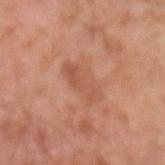Findings:
* biopsy status — catalogued during a skin exam; not biopsied
* TBP lesion metrics — an area of roughly 9 mm², an eccentricity of roughly 0.9, and two-axis asymmetry of about 0.25
* acquisition — ~15 mm tile from a whole-body skin photo
* lighting — white-light
* body site — the arm
* subject — male, about 75 years old